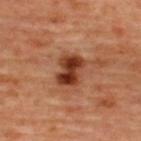| field | value |
|---|---|
| biopsy status | imaged on a skin check; not biopsied |
| subject | female, aged 43 to 47 |
| illumination | cross-polarized illumination |
| diameter | about 4 mm |
| automated metrics | an outline eccentricity of about 0.7 (0 = round, 1 = elongated) and a shape-asymmetry score of about 0.2 (0 = symmetric); border irregularity of about 2.5 on a 0–10 scale, a within-lesion color-variation index near 6/10, and a peripheral color-asymmetry measure near 2.5; a nevus-likeness score of about 90/100 and a lesion-detection confidence of about 100/100 |
| body site | the upper back |
| image | ~15 mm tile from a whole-body skin photo |